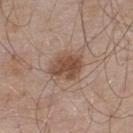workup: catalogued during a skin exam; not biopsied
acquisition: ~15 mm crop, total-body skin-cancer survey
patient: male, aged approximately 55
image-analysis metrics: a footprint of about 10 mm² and two-axis asymmetry of about 0.15; an average lesion color of about L≈49 a*≈18 b*≈27 (CIELAB), roughly 11 lightness units darker than nearby skin, and a lesion-to-skin contrast of about 8.5 (normalized; higher = more distinct); a border-irregularity rating of about 1.5/10, internal color variation of about 3.5 on a 0–10 scale, and a peripheral color-asymmetry measure near 1; a nevus-likeness score of about 80/100 and a lesion-detection confidence of about 100/100
diameter: ≈4 mm
location: the upper back